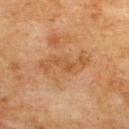The lesion was photographed on a routine skin check and not biopsied; there is no pathology result. A male patient, aged around 75. Located on the upper back. A 15 mm close-up tile from a total-body photography series done for melanoma screening. Longest diameter approximately 6 mm. This is a cross-polarized tile.